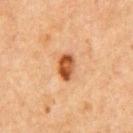Case summary:
– notes · catalogued during a skin exam; not biopsied
– TBP lesion metrics · a symmetry-axis asymmetry near 0.15; an automated nevus-likeness rating near 95 out of 100 and a detector confidence of about 100 out of 100 that the crop contains a lesion
– lesion size · about 3.5 mm
– illumination · cross-polarized
– subject · male, aged around 65
– imaging modality · ~15 mm tile from a whole-body skin photo
– anatomic site · the chest